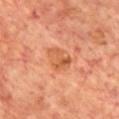From the chest. A 15 mm close-up extracted from a 3D total-body photography capture. Imaged with cross-polarized lighting. A male patient aged 68 to 72. Approximately 3.5 mm at its widest.